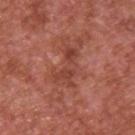<case>
<biopsy_status>not biopsied; imaged during a skin examination</biopsy_status>
<site>upper back</site>
<patient>
  <sex>male</sex>
  <age_approx>65</age_approx>
</patient>
<image>
  <source>total-body photography crop</source>
  <field_of_view_mm>15</field_of_view_mm>
</image>
<lighting>white-light</lighting>
</case>